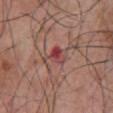Q: Was this lesion biopsied?
A: total-body-photography surveillance lesion; no biopsy
Q: Illumination type?
A: white-light illumination
Q: Patient demographics?
A: male, aged around 70
Q: How was this image acquired?
A: total-body-photography crop, ~15 mm field of view
Q: Where on the body is the lesion?
A: the chest
Q: How large is the lesion?
A: about 3.5 mm